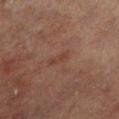Image and clinical context: The total-body-photography lesion software estimated an outline eccentricity of about 0.85 (0 = round, 1 = elongated) and two-axis asymmetry of about 0.3. It also reported an average lesion color of about L≈32 a*≈17 b*≈22 (CIELAB) and roughly 4 lightness units darker than nearby skin. The analysis additionally found border irregularity of about 3 on a 0–10 scale, internal color variation of about 0.5 on a 0–10 scale, and a peripheral color-asymmetry measure near 0. And it measured a nevus-likeness score of about 0/100 and lesion-presence confidence of about 100/100. Captured under cross-polarized illumination. Longest diameter approximately 2.5 mm. A close-up tile cropped from a whole-body skin photograph, about 15 mm across. A male subject, aged 68–72. From the left lower leg.